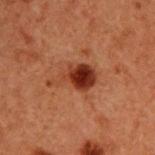– workup · no biopsy performed (imaged during a skin exam)
– illumination · cross-polarized
– subject · male, aged around 60
– site · the upper back
– acquisition · ~15 mm tile from a whole-body skin photo